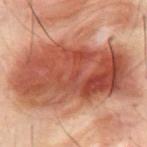Findings:
– biopsy status: no biopsy performed (imaged during a skin exam)
– subject: male, in their 30s
– lighting: cross-polarized illumination
– body site: the abdomen
– lesion diameter: about 13.5 mm
– image source: total-body-photography crop, ~15 mm field of view